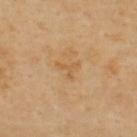Findings:
• biopsy status · no biopsy performed (imaged during a skin exam)
• size · about 2.5 mm
• automated lesion analysis · a border-irregularity rating of about 7.5/10 and radial color variation of about 0; an automated nevus-likeness rating near 0 out of 100 and a detector confidence of about 100 out of 100 that the crop contains a lesion
• illumination · cross-polarized
• imaging modality · 15 mm crop, total-body photography
• subject · male, aged 53–57
• anatomic site · the upper back